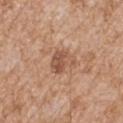Notes:
• workup — no biopsy performed (imaged during a skin exam)
• patient — male, aged 63–67
• image — ~15 mm crop, total-body skin-cancer survey
• illumination — white-light illumination
• lesion diameter — about 3 mm
• body site — the right upper arm